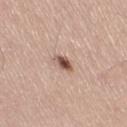This lesion was catalogued during total-body skin photography and was not selected for biopsy. This image is a 15 mm lesion crop taken from a total-body photograph. A male subject, aged 58 to 62. Imaged with white-light lighting. The lesion is on the leg.